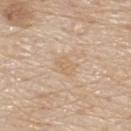Findings:
– biopsy status: catalogued during a skin exam; not biopsied
– tile lighting: white-light
– anatomic site: the upper back
– patient: male, approximately 80 years of age
– lesion size: ~2.5 mm (longest diameter)
– imaging modality: 15 mm crop, total-body photography
– automated metrics: an area of roughly 4 mm² and an eccentricity of roughly 0.75; an automated nevus-likeness rating near 0 out of 100